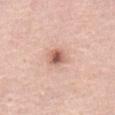Clinical impression:
This lesion was catalogued during total-body skin photography and was not selected for biopsy.